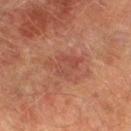follow-up = no biopsy performed (imaged during a skin exam)
site = the left lower leg
automated lesion analysis = a symmetry-axis asymmetry near 0.5; border irregularity of about 6.5 on a 0–10 scale, internal color variation of about 3.5 on a 0–10 scale, and peripheral color asymmetry of about 1.5; a classifier nevus-likeness of about 0/100
subject = male, roughly 75 years of age
lesion size = about 4.5 mm
illumination = cross-polarized
imaging modality = ~15 mm tile from a whole-body skin photo